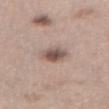Q: Was a biopsy performed?
A: catalogued during a skin exam; not biopsied
Q: Lesion size?
A: ≈3.5 mm
Q: What lighting was used for the tile?
A: white-light
Q: Automated lesion metrics?
A: a lesion area of about 6.5 mm² and a symmetry-axis asymmetry near 0.15; border irregularity of about 1.5 on a 0–10 scale and a within-lesion color-variation index near 5.5/10
Q: What are the patient's age and sex?
A: female, approximately 40 years of age
Q: Where on the body is the lesion?
A: the abdomen
Q: What is the imaging modality?
A: 15 mm crop, total-body photography